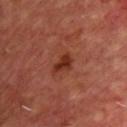Imaged during a routine full-body skin examination; the lesion was not biopsied and no histopathology is available.